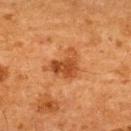Case summary:
- workup · no biopsy performed (imaged during a skin exam)
- automated metrics · internal color variation of about 4 on a 0–10 scale and peripheral color asymmetry of about 1.5
- location · the upper back
- subject · male, in their mid-60s
- image · ~15 mm crop, total-body skin-cancer survey